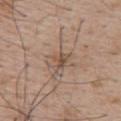follow-up = no biopsy performed (imaged during a skin exam) | tile lighting = white-light illumination | patient = male, approximately 65 years of age | site = the upper back | imaging modality = ~15 mm crop, total-body skin-cancer survey | automated lesion analysis = a footprint of about 3.5 mm² and a symmetry-axis asymmetry near 0.45; a lesion color around L≈50 a*≈16 b*≈27 in CIELAB, a lesion–skin lightness drop of about 8, and a normalized lesion–skin contrast near 6.5; an automated nevus-likeness rating near 0 out of 100 and lesion-presence confidence of about 95/100 | diameter = about 2.5 mm.